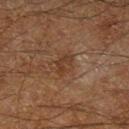* follow-up: total-body-photography surveillance lesion; no biopsy
* lesion size: ≈3 mm
* image-analysis metrics: an area of roughly 4.5 mm² and two-axis asymmetry of about 0.25; a border-irregularity rating of about 2.5/10 and a peripheral color-asymmetry measure near 1
* acquisition: ~15 mm crop, total-body skin-cancer survey
* anatomic site: the left lower leg
* subject: male, approximately 60 years of age